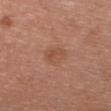The lesion was tiled from a total-body skin photograph and was not biopsied. A female patient, aged 38 to 42. A lesion tile, about 15 mm wide, cut from a 3D total-body photograph. The lesion is located on the chest.